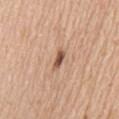  biopsy_status: not biopsied; imaged during a skin examination
  lighting: white-light
  automated_metrics:
    eccentricity: 0.9
    shape_asymmetry: 0.3
    cielab_L: 53
    cielab_a: 22
    cielab_b: 30
    vs_skin_darker_L: 15.0
    border_irregularity_0_10: 3.0
    peripheral_color_asymmetry: 0.0
    nevus_likeness_0_100: 95
    lesion_detection_confidence_0_100: 100
  site: front of the torso
  patient:
    sex: male
    age_approx: 70
  image:
    source: total-body photography crop
    field_of_view_mm: 15